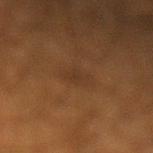Impression: The lesion was tiled from a total-body skin photograph and was not biopsied. Image and clinical context: The lesion is on the left lower leg. Cropped from a total-body skin-imaging series; the visible field is about 15 mm. A male subject roughly 60 years of age.